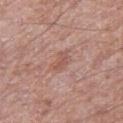biopsy status: total-body-photography surveillance lesion; no biopsy
image source: ~15 mm crop, total-body skin-cancer survey
tile lighting: white-light illumination
site: the right thigh
subject: male, aged around 65